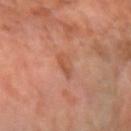image:
  source: total-body photography crop
  field_of_view_mm: 15
patient:
  sex: female
  age_approx: 65
lighting: cross-polarized
lesion_size:
  long_diameter_mm_approx: 3.0
site: left forearm